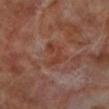| field | value |
|---|---|
| follow-up | total-body-photography surveillance lesion; no biopsy |
| automated metrics | an area of roughly 7 mm², an outline eccentricity of about 0.75 (0 = round, 1 = elongated), and two-axis asymmetry of about 0.45; about 6 CIELAB-L* units darker than the surrounding skin and a normalized lesion–skin contrast near 6; a border-irregularity index near 4.5/10, internal color variation of about 2.5 on a 0–10 scale, and peripheral color asymmetry of about 1; an automated nevus-likeness rating near 0 out of 100 and a lesion-detection confidence of about 100/100 |
| site | the left lower leg |
| acquisition | total-body-photography crop, ~15 mm field of view |
| lighting | cross-polarized |
| patient | male, aged around 70 |
| lesion diameter | about 4 mm |